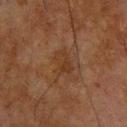Q: Was a biopsy performed?
A: imaged on a skin check; not biopsied
Q: Patient demographics?
A: male, in their mid-60s
Q: Lesion location?
A: the upper back
Q: What kind of image is this?
A: 15 mm crop, total-body photography
Q: Automated lesion metrics?
A: a lesion area of about 6 mm², an outline eccentricity of about 0.7 (0 = round, 1 = elongated), and a symmetry-axis asymmetry near 0.25; a classifier nevus-likeness of about 0/100
Q: What is the lesion's diameter?
A: about 3.5 mm